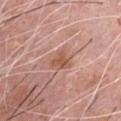Impression:
The lesion was tiled from a total-body skin photograph and was not biopsied.
Acquisition and patient details:
A male subject aged 58 to 62. The lesion is located on the chest. A 15 mm close-up extracted from a 3D total-body photography capture. Automated tile analysis of the lesion measured a footprint of about 6.5 mm², a shape eccentricity near 0.7, and a symmetry-axis asymmetry near 0.35. The software also gave a mean CIELAB color near L≈56 a*≈22 b*≈29, about 8 CIELAB-L* units darker than the surrounding skin, and a normalized lesion–skin contrast near 6.5. The analysis additionally found a border-irregularity rating of about 3.5/10, internal color variation of about 2.5 on a 0–10 scale, and a peripheral color-asymmetry measure near 1. It also reported a classifier nevus-likeness of about 0/100 and a detector confidence of about 100 out of 100 that the crop contains a lesion.